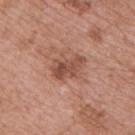biopsy status — imaged on a skin check; not biopsied
image — ~15 mm crop, total-body skin-cancer survey
site — the back
patient — male, aged around 65
lesion diameter — ~4 mm (longest diameter)
illumination — white-light illumination